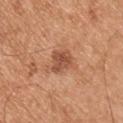| key | value |
|---|---|
| biopsy status | no biopsy performed (imaged during a skin exam) |
| imaging modality | ~15 mm crop, total-body skin-cancer survey |
| image-analysis metrics | roughly 11 lightness units darker than nearby skin and a normalized border contrast of about 7.5 |
| anatomic site | the right upper arm |
| illumination | white-light |
| subject | male, about 55 years old |
| lesion size | ~3 mm (longest diameter) |